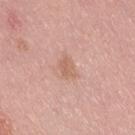- subject · male, approximately 40 years of age
- imaging modality · ~15 mm tile from a whole-body skin photo
- location · the lower back